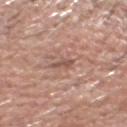Q: Is there a histopathology result?
A: imaged on a skin check; not biopsied
Q: What is the lesion's diameter?
A: about 3 mm
Q: Patient demographics?
A: male, aged 63–67
Q: Lesion location?
A: the head or neck
Q: What kind of image is this?
A: ~15 mm tile from a whole-body skin photo